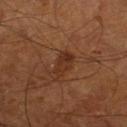Q: Was this lesion biopsied?
A: catalogued during a skin exam; not biopsied
Q: What did automated image analysis measure?
A: a lesion area of about 5 mm², a shape eccentricity near 0.8, and a shape-asymmetry score of about 0.25 (0 = symmetric); a within-lesion color-variation index near 3.5/10 and radial color variation of about 1.5; a classifier nevus-likeness of about 15/100 and a detector confidence of about 100 out of 100 that the crop contains a lesion
Q: Lesion location?
A: the left leg
Q: How was this image acquired?
A: 15 mm crop, total-body photography
Q: What are the patient's age and sex?
A: male, approximately 65 years of age
Q: How large is the lesion?
A: about 3 mm